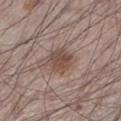About 3 mm across.
The subject is a male aged around 60.
A roughly 15 mm field-of-view crop from a total-body skin photograph.
On the left lower leg.
An algorithmic analysis of the crop reported a lesion color around L≈47 a*≈17 b*≈23 in CIELAB and roughly 10 lightness units darker than nearby skin. It also reported a border-irregularity index near 2/10, internal color variation of about 3.5 on a 0–10 scale, and peripheral color asymmetry of about 1.5. It also reported an automated nevus-likeness rating near 80 out of 100 and a detector confidence of about 100 out of 100 that the crop contains a lesion.